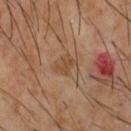Case summary:
- notes — catalogued during a skin exam; not biopsied
- body site — the chest
- patient — male, in their 60s
- acquisition — 15 mm crop, total-body photography
- diameter — ~3 mm (longest diameter)